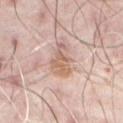subject: male, aged 48–52
lesion size: about 4 mm
acquisition: total-body-photography crop, ~15 mm field of view
location: the abdomen